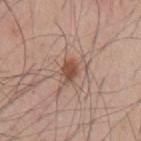biopsy status: total-body-photography surveillance lesion; no biopsy
lighting: white-light illumination
patient: male, aged 53–57
diameter: ≈2.5 mm
location: the chest
acquisition: ~15 mm tile from a whole-body skin photo
automated metrics: a lesion area of about 3.5 mm², an outline eccentricity of about 0.7 (0 = round, 1 = elongated), and a symmetry-axis asymmetry near 0.15; a classifier nevus-likeness of about 95/100 and a detector confidence of about 100 out of 100 that the crop contains a lesion